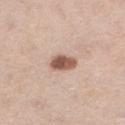No biopsy was performed on this lesion — it was imaged during a full skin examination and was not determined to be concerning.
The lesion is located on the right lower leg.
Cropped from a whole-body photographic skin survey; the tile spans about 15 mm.
A female patient aged around 65.